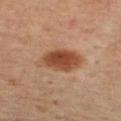workup = catalogued during a skin exam; not biopsied
image-analysis metrics = a lesion color around L≈48 a*≈23 b*≈33 in CIELAB, roughly 13 lightness units darker than nearby skin, and a normalized lesion–skin contrast near 9.5; a border-irregularity index near 2/10, a within-lesion color-variation index near 4.5/10, and peripheral color asymmetry of about 1.5
subject = male, aged around 65
body site = the right thigh
imaging modality = 15 mm crop, total-body photography